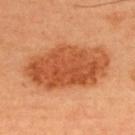Clinical summary:
An algorithmic analysis of the crop reported roughly 13 lightness units darker than nearby skin and a normalized border contrast of about 9. The analysis additionally found a classifier nevus-likeness of about 100/100 and a detector confidence of about 100 out of 100 that the crop contains a lesion. A roughly 15 mm field-of-view crop from a total-body skin photograph. The patient is a male aged approximately 35. From the upper back. The recorded lesion diameter is about 10 mm. The tile uses cross-polarized illumination.